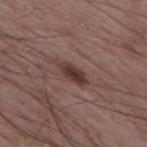biopsy_status: not biopsied; imaged during a skin examination
lesion_size:
  long_diameter_mm_approx: 3.5
patient:
  sex: male
  age_approx: 55
site: upper back
image:
  source: total-body photography crop
  field_of_view_mm: 15